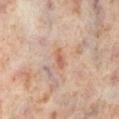A female subject approximately 50 years of age.
Approximately 2.5 mm at its widest.
A lesion tile, about 15 mm wide, cut from a 3D total-body photograph.
This is a cross-polarized tile.
On the right lower leg.
The total-body-photography lesion software estimated a footprint of about 2.5 mm², an eccentricity of roughly 0.9, and a shape-asymmetry score of about 0.3 (0 = symmetric). The software also gave about 8 CIELAB-L* units darker than the surrounding skin and a normalized lesion–skin contrast near 6. It also reported a nevus-likeness score of about 0/100 and lesion-presence confidence of about 100/100.